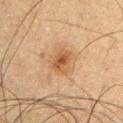Clinical summary:
On the front of the torso. Cropped from a total-body skin-imaging series; the visible field is about 15 mm. Automated image analysis of the tile measured a border-irregularity rating of about 2.5/10 and a peripheral color-asymmetry measure near 1.5. Measured at roughly 3 mm in maximum diameter. A male patient aged 33–37. The tile uses cross-polarized illumination.